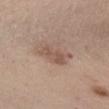Case summary:
- follow-up: catalogued during a skin exam; not biopsied
- anatomic site: the chest
- illumination: white-light illumination
- patient: female, aged around 65
- image source: total-body-photography crop, ~15 mm field of view